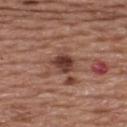No biopsy was performed on this lesion — it was imaged during a full skin examination and was not determined to be concerning.
An algorithmic analysis of the crop reported an area of roughly 6 mm², an eccentricity of roughly 0.55, and a symmetry-axis asymmetry near 0.25. The software also gave a mean CIELAB color near L≈40 a*≈22 b*≈25, roughly 12 lightness units darker than nearby skin, and a normalized lesion–skin contrast near 10. The analysis additionally found a border-irregularity index near 2.5/10 and a color-variation rating of about 5.5/10.
Measured at roughly 3 mm in maximum diameter.
A roughly 15 mm field-of-view crop from a total-body skin photograph.
The subject is a male in their mid- to late 50s.
The lesion is located on the upper back.